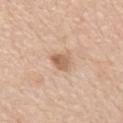biopsy status = no biopsy performed (imaged during a skin exam); image source = 15 mm crop, total-body photography; body site = the chest; subject = male, approximately 75 years of age.